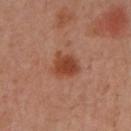Clinical impression: The lesion was tiled from a total-body skin photograph and was not biopsied.